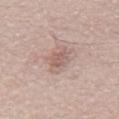| key | value |
|---|---|
| biopsy status | no biopsy performed (imaged during a skin exam) |
| lesion size | ~3.5 mm (longest diameter) |
| patient | male, aged around 65 |
| illumination | white-light illumination |
| location | the abdomen |
| automated lesion analysis | a footprint of about 5.5 mm², an eccentricity of roughly 0.75, and a symmetry-axis asymmetry near 0.3; a mean CIELAB color near L≈59 a*≈18 b*≈23, roughly 9 lightness units darker than nearby skin, and a normalized border contrast of about 6; a border-irregularity rating of about 3.5/10 and internal color variation of about 2.5 on a 0–10 scale |
| acquisition | ~15 mm tile from a whole-body skin photo |